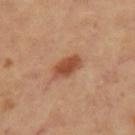workup = catalogued during a skin exam; not biopsied | patient = female, approximately 60 years of age | size = about 4 mm | body site = the left leg | imaging modality = ~15 mm crop, total-body skin-cancer survey.